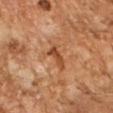Q: Was this lesion biopsied?
A: total-body-photography surveillance lesion; no biopsy
Q: Who is the patient?
A: male, aged 58 to 62
Q: Where on the body is the lesion?
A: the right forearm
Q: What did automated image analysis measure?
A: border irregularity of about 5 on a 0–10 scale, internal color variation of about 3 on a 0–10 scale, and a peripheral color-asymmetry measure near 1
Q: What is the lesion's diameter?
A: about 3.5 mm
Q: What lighting was used for the tile?
A: cross-polarized illumination
Q: What kind of image is this?
A: ~15 mm tile from a whole-body skin photo